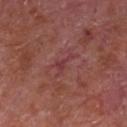| key | value |
|---|---|
| workup | imaged on a skin check; not biopsied |
| anatomic site | the front of the torso |
| tile lighting | white-light illumination |
| image-analysis metrics | a border-irregularity rating of about 7.5/10, a color-variation rating of about 0/10, and radial color variation of about 0 |
| subject | male, aged 63 to 67 |
| imaging modality | 15 mm crop, total-body photography |
| lesion diameter | ~3 mm (longest diameter) |